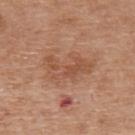<record>
  <biopsy_status>not biopsied; imaged during a skin examination</biopsy_status>
  <site>back</site>
  <patient>
    <sex>female</sex>
    <age_approx>75</age_approx>
  </patient>
  <automated_metrics>
    <area_mm2_approx>14.0</area_mm2_approx>
    <eccentricity>0.85</eccentricity>
    <cielab_L>52</cielab_L>
    <cielab_a>22</cielab_a>
    <cielab_b>32</cielab_b>
    <vs_skin_darker_L>8.0</vs_skin_darker_L>
    <border_irregularity_0_10>5.0</border_irregularity_0_10>
    <color_variation_0_10>3.5</color_variation_0_10>
    <peripheral_color_asymmetry>1.0</peripheral_color_asymmetry>
    <nevus_likeness_0_100>0</nevus_likeness_0_100>
    <lesion_detection_confidence_0_100>100</lesion_detection_confidence_0_100>
  </automated_metrics>
  <lesion_size>
    <long_diameter_mm_approx>5.5</long_diameter_mm_approx>
  </lesion_size>
  <image>
    <source>total-body photography crop</source>
    <field_of_view_mm>15</field_of_view_mm>
  </image>
  <lighting>white-light</lighting>
</record>